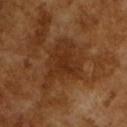Notes:
• notes — catalogued during a skin exam; not biopsied
• imaging modality — total-body-photography crop, ~15 mm field of view
• size — ≈7 mm
• patient — male, aged 63 to 67
• tile lighting — cross-polarized